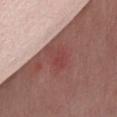Recorded during total-body skin imaging; not selected for excision or biopsy. A region of skin cropped from a whole-body photographic capture, roughly 15 mm wide. A female patient, approximately 50 years of age. The lesion is on the chest. The total-body-photography lesion software estimated an area of roughly 6 mm² and an outline eccentricity of about 0.7 (0 = round, 1 = elongated). It also reported a border-irregularity index near 3/10 and internal color variation of about 3 on a 0–10 scale. The analysis additionally found a classifier nevus-likeness of about 0/100 and a lesion-detection confidence of about 90/100.